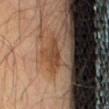No biopsy was performed on this lesion — it was imaged during a full skin examination and was not determined to be concerning. The lesion is on the front of the torso. Automated image analysis of the tile measured a shape eccentricity near 0.9 and a shape-asymmetry score of about 0.4 (0 = symmetric). The software also gave a lesion color around L≈39 a*≈18 b*≈29 in CIELAB. And it measured a detector confidence of about 70 out of 100 that the crop contains a lesion. A male subject approximately 60 years of age. A lesion tile, about 15 mm wide, cut from a 3D total-body photograph.